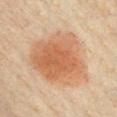Assessment:
Imaged during a routine full-body skin examination; the lesion was not biopsied and no histopathology is available.
Image and clinical context:
A male patient, aged 38–42. A close-up tile cropped from a whole-body skin photograph, about 15 mm across. The lesion is located on the chest. About 8 mm across.